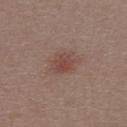Case summary:
- notes — catalogued during a skin exam; not biopsied
- patient — female, in their 30s
- location — the back
- size — ~3 mm (longest diameter)
- image — 15 mm crop, total-body photography
- automated metrics — a footprint of about 6 mm², an eccentricity of roughly 0.55, and a symmetry-axis asymmetry near 0.25; a mean CIELAB color near L≈45 a*≈20 b*≈24, roughly 8 lightness units darker than nearby skin, and a lesion-to-skin contrast of about 6 (normalized; higher = more distinct)